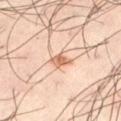This lesion was catalogued during total-body skin photography and was not selected for biopsy. The lesion is on the left thigh. A male subject, aged around 40. Cropped from a whole-body photographic skin survey; the tile spans about 15 mm.